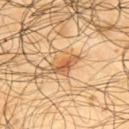Captured during whole-body skin photography for melanoma surveillance; the lesion was not biopsied. Captured under cross-polarized illumination. From the upper back. A male subject aged 63–67. Cropped from a whole-body photographic skin survey; the tile spans about 15 mm.